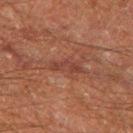Q: Is there a histopathology result?
A: catalogued during a skin exam; not biopsied
Q: Patient demographics?
A: male, approximately 60 years of age
Q: How was this image acquired?
A: ~15 mm tile from a whole-body skin photo
Q: Illumination type?
A: cross-polarized
Q: Lesion size?
A: about 3.5 mm
Q: What did automated image analysis measure?
A: an automated nevus-likeness rating near 0 out of 100 and a lesion-detection confidence of about 85/100
Q: Where on the body is the lesion?
A: the left thigh